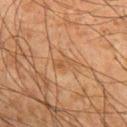Recorded during total-body skin imaging; not selected for excision or biopsy. The lesion is located on the right upper arm. A male subject in their mid- to late 60s. About 3 mm across. A 15 mm close-up tile from a total-body photography series done for melanoma screening.